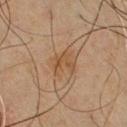Clinical impression: Captured during whole-body skin photography for melanoma surveillance; the lesion was not biopsied. Background: Automated image analysis of the tile measured a lesion area of about 4.5 mm² and a symmetry-axis asymmetry near 0.55. The software also gave a lesion color around L≈40 a*≈16 b*≈29 in CIELAB, a lesion–skin lightness drop of about 6, and a lesion-to-skin contrast of about 6 (normalized; higher = more distinct). And it measured border irregularity of about 6.5 on a 0–10 scale, a within-lesion color-variation index near 3/10, and radial color variation of about 1.5. Captured under cross-polarized illumination. Approximately 2.5 mm at its widest. A male patient roughly 45 years of age. A 15 mm crop from a total-body photograph taken for skin-cancer surveillance. Located on the chest.